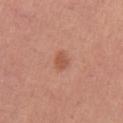biopsy status: total-body-photography surveillance lesion; no biopsy
imaging modality: total-body-photography crop, ~15 mm field of view
tile lighting: white-light
TBP lesion metrics: an average lesion color of about L≈54 a*≈26 b*≈32 (CIELAB) and a lesion-to-skin contrast of about 6 (normalized; higher = more distinct); a border-irregularity rating of about 2.5/10 and a peripheral color-asymmetry measure near 0.5
subject: male, about 45 years old
lesion size: ≈2.5 mm
site: the mid back